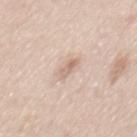follow-up = imaged on a skin check; not biopsied | lesion size = ~2.5 mm (longest diameter) | tile lighting = white-light | acquisition = 15 mm crop, total-body photography | site = the mid back | patient = male, in their mid- to late 40s.